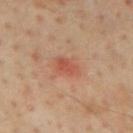Recorded during total-body skin imaging; not selected for excision or biopsy. The lesion is on the mid back. The tile uses cross-polarized illumination. A male subject in their mid- to late 50s. A 15 mm close-up tile from a total-body photography series done for melanoma screening. Longest diameter approximately 3 mm.